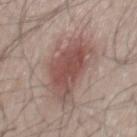The lesion was tiled from a total-body skin photograph and was not biopsied.
A region of skin cropped from a whole-body photographic capture, roughly 15 mm wide.
Located on the back.
Longest diameter approximately 8 mm.
A male subject, in their 50s.
This is a white-light tile.